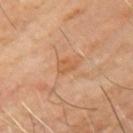Clinical summary: On the left upper arm. Captured under cross-polarized illumination. A 15 mm close-up extracted from a 3D total-body photography capture. A male subject, aged 63–67. An algorithmic analysis of the crop reported a lesion area of about 3.5 mm² and a shape-asymmetry score of about 0.35 (0 = symmetric). And it measured a mean CIELAB color near L≈55 a*≈22 b*≈37, a lesion–skin lightness drop of about 7, and a normalized border contrast of about 5.5. And it measured an automated nevus-likeness rating near 0 out of 100 and lesion-presence confidence of about 100/100.